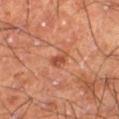Assessment:
This lesion was catalogued during total-body skin photography and was not selected for biopsy.
Acquisition and patient details:
The tile uses cross-polarized illumination. Longest diameter approximately 2.5 mm. The lesion is on the right thigh. An algorithmic analysis of the crop reported a color-variation rating of about 1.5/10 and a peripheral color-asymmetry measure near 0.5. This image is a 15 mm lesion crop taken from a total-body photograph. A male patient, about 60 years old.